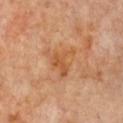Imaged during a routine full-body skin examination; the lesion was not biopsied and no histopathology is available. Automated image analysis of the tile measured an area of roughly 9.5 mm² and a shape-asymmetry score of about 0.5 (0 = symmetric). The recorded lesion diameter is about 4.5 mm. A female subject, aged around 65. This is a cross-polarized tile. From the chest. A 15 mm close-up extracted from a 3D total-body photography capture.